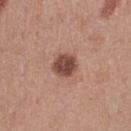Recorded during total-body skin imaging; not selected for excision or biopsy. Approximately 3 mm at its widest. This image is a 15 mm lesion crop taken from a total-body photograph. A female patient, aged 38–42. Automated tile analysis of the lesion measured a nevus-likeness score of about 55/100 and lesion-presence confidence of about 100/100. The lesion is on the right thigh. Captured under white-light illumination.